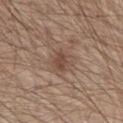notes = total-body-photography surveillance lesion; no biopsy
site = the front of the torso
TBP lesion metrics = an average lesion color of about L≈48 a*≈17 b*≈25 (CIELAB), roughly 8 lightness units darker than nearby skin, and a normalized lesion–skin contrast near 6; border irregularity of about 2 on a 0–10 scale, a within-lesion color-variation index near 3/10, and radial color variation of about 1; a nevus-likeness score of about 5/100 and lesion-presence confidence of about 100/100
patient = male, approximately 80 years of age
tile lighting = white-light illumination
acquisition = 15 mm crop, total-body photography
size = ≈3 mm